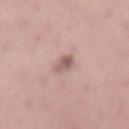{
  "biopsy_status": "not biopsied; imaged during a skin examination",
  "image": {
    "source": "total-body photography crop",
    "field_of_view_mm": 15
  },
  "patient": {
    "sex": "female",
    "age_approx": 65
  },
  "site": "mid back"
}